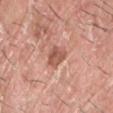A lesion tile, about 15 mm wide, cut from a 3D total-body photograph. From the front of the torso. A male patient, aged approximately 40.